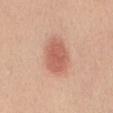Impression:
The lesion was tiled from a total-body skin photograph and was not biopsied.
Acquisition and patient details:
Captured under white-light illumination. An algorithmic analysis of the crop reported an area of roughly 12 mm², an outline eccentricity of about 0.8 (0 = round, 1 = elongated), and two-axis asymmetry of about 0.15. The analysis additionally found a lesion color around L≈60 a*≈26 b*≈30 in CIELAB and about 12 CIELAB-L* units darker than the surrounding skin. The software also gave lesion-presence confidence of about 100/100. Longest diameter approximately 5 mm. On the mid back. A female patient, in their 50s. A roughly 15 mm field-of-view crop from a total-body skin photograph.